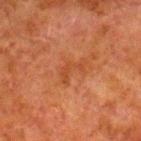workup — total-body-photography surveillance lesion; no biopsy
location — the right lower leg
illumination — cross-polarized illumination
lesion diameter — ≈3.5 mm
image source — total-body-photography crop, ~15 mm field of view
subject — male, aged 78–82
image-analysis metrics — an area of roughly 4 mm² and two-axis asymmetry of about 0.8; lesion-presence confidence of about 100/100